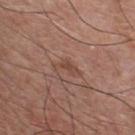| field | value |
|---|---|
| location | the chest |
| lesion diameter | about 3.5 mm |
| image | 15 mm crop, total-body photography |
| patient | male, aged 73–77 |
| lighting | white-light |